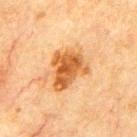Part of a total-body skin-imaging series; this lesion was reviewed on a skin check and was not flagged for biopsy.